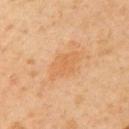Captured during whole-body skin photography for melanoma surveillance; the lesion was not biopsied. The recorded lesion diameter is about 3.5 mm. Cropped from a total-body skin-imaging series; the visible field is about 15 mm. Imaged with cross-polarized lighting. The lesion is located on the left upper arm. The subject is a female in their 40s.